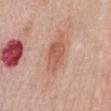<tbp_lesion>
  <biopsy_status>not biopsied; imaged during a skin examination</biopsy_status>
  <lesion_size>
    <long_diameter_mm_approx>4.5</long_diameter_mm_approx>
  </lesion_size>
  <patient>
    <sex>female</sex>
    <age_approx>65</age_approx>
  </patient>
  <site>chest</site>
  <image>
    <source>total-body photography crop</source>
    <field_of_view_mm>15</field_of_view_mm>
  </image>
  <automated_metrics>
    <border_irregularity_0_10>2.0</border_irregularity_0_10>
    <peripheral_color_asymmetry>1.0</peripheral_color_asymmetry>
    <nevus_likeness_0_100>100</nevus_likeness_0_100>
  </automated_metrics>
  <lighting>white-light</lighting>
</tbp_lesion>